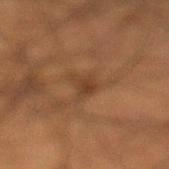{
  "biopsy_status": "not biopsied; imaged during a skin examination",
  "patient": {
    "sex": "male",
    "age_approx": 50
  },
  "site": "left lower leg",
  "lesion_size": {
    "long_diameter_mm_approx": 2.5
  },
  "image": {
    "source": "total-body photography crop",
    "field_of_view_mm": 15
  }
}